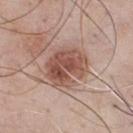Q: Was a biopsy performed?
A: no biopsy performed (imaged during a skin exam)
Q: What is the imaging modality?
A: ~15 mm crop, total-body skin-cancer survey
Q: Patient demographics?
A: male, aged 53–57
Q: Automated lesion metrics?
A: an area of roughly 17 mm² and a shape eccentricity near 0.6; an average lesion color of about L≈52 a*≈21 b*≈26 (CIELAB), about 13 CIELAB-L* units darker than the surrounding skin, and a normalized lesion–skin contrast near 9; a nevus-likeness score of about 75/100 and a lesion-detection confidence of about 100/100
Q: Lesion location?
A: the chest
Q: What is the lesion's diameter?
A: ≈5 mm
Q: What lighting was used for the tile?
A: white-light illumination